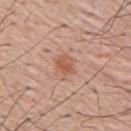workup = imaged on a skin check; not biopsied
patient = male, in their mid- to late 50s
imaging modality = 15 mm crop, total-body photography
tile lighting = white-light illumination
lesion size = ~3 mm (longest diameter)